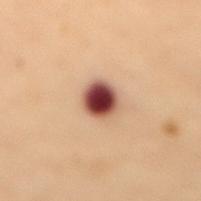{
  "biopsy_status": "not biopsied; imaged during a skin examination",
  "lesion_size": {
    "long_diameter_mm_approx": 3.0
  },
  "patient": {
    "sex": "female",
    "age_approx": 50
  },
  "automated_metrics": {
    "border_irregularity_0_10": 1.0,
    "color_variation_0_10": 6.5,
    "nevus_likeness_0_100": 95,
    "lesion_detection_confidence_0_100": 100
  },
  "image": {
    "source": "total-body photography crop",
    "field_of_view_mm": 15
  },
  "site": "mid back",
  "lighting": "cross-polarized"
}